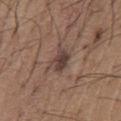- biopsy status — catalogued during a skin exam; not biopsied
- body site — the chest
- diameter — ~4 mm (longest diameter)
- image source — ~15 mm crop, total-body skin-cancer survey
- patient — male, aged around 65
- tile lighting — white-light